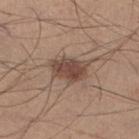This lesion was catalogued during total-body skin photography and was not selected for biopsy.